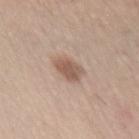Imaged during a routine full-body skin examination; the lesion was not biopsied and no histopathology is available. Automated tile analysis of the lesion measured a shape-asymmetry score of about 0.25 (0 = symmetric). It also reported roughly 11 lightness units darker than nearby skin and a normalized lesion–skin contrast near 7.5. Cropped from a total-body skin-imaging series; the visible field is about 15 mm. Approximately 3 mm at its widest. The lesion is located on the abdomen. Imaged with white-light lighting. The subject is a female aged 38–42.